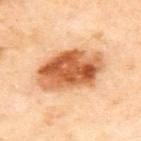Assessment: Part of a total-body skin-imaging series; this lesion was reviewed on a skin check and was not flagged for biopsy. Context: The lesion's longest dimension is about 7.5 mm. The lesion is on the upper back. A female patient about 60 years old. The tile uses cross-polarized illumination. A lesion tile, about 15 mm wide, cut from a 3D total-body photograph.